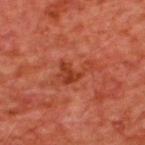Background: A close-up tile cropped from a whole-body skin photograph, about 15 mm across. Longest diameter approximately 4 mm. The patient is a male aged 63–67. Captured under cross-polarized illumination. On the upper back. The lesion-visualizer software estimated a lesion area of about 6.5 mm², an eccentricity of roughly 0.85, and a symmetry-axis asymmetry near 0.55. The analysis additionally found a border-irregularity rating of about 6.5/10, internal color variation of about 3 on a 0–10 scale, and radial color variation of about 1.